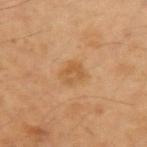Findings:
* follow-up · catalogued during a skin exam; not biopsied
* image-analysis metrics · a footprint of about 6 mm² and an eccentricity of roughly 0.5; roughly 7 lightness units darker than nearby skin; a border-irregularity index near 2.5/10, a color-variation rating of about 3/10, and peripheral color asymmetry of about 1
* size · ≈3 mm
* patient · male, roughly 50 years of age
* anatomic site · the left upper arm
* image source · ~15 mm tile from a whole-body skin photo
* illumination · cross-polarized